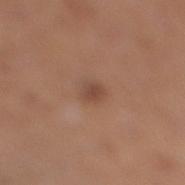Clinical impression: This lesion was catalogued during total-body skin photography and was not selected for biopsy. Acquisition and patient details: A 15 mm close-up tile from a total-body photography series done for melanoma screening. Approximately 2.5 mm at its widest. Automated image analysis of the tile measured a footprint of about 3.5 mm², a shape eccentricity near 0.7, and a symmetry-axis asymmetry near 0.2. It also reported a normalized border contrast of about 6. A female subject, about 40 years old. Located on the leg.